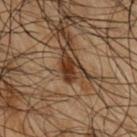notes: catalogued during a skin exam; not biopsied
image source: ~15 mm tile from a whole-body skin photo
illumination: cross-polarized illumination
patient: male, aged 48–52
automated lesion analysis: a lesion-detection confidence of about 100/100
location: the arm